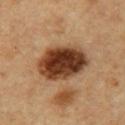The lesion was photographed on a routine skin check and not biopsied; there is no pathology result.
A male subject, about 55 years old.
The lesion is on the arm.
A 15 mm close-up extracted from a 3D total-body photography capture.